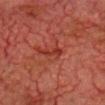biopsy status: total-body-photography surveillance lesion; no biopsy.